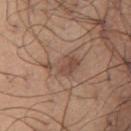Impression:
The lesion was photographed on a routine skin check and not biopsied; there is no pathology result.
Clinical summary:
Measured at roughly 4 mm in maximum diameter. The lesion is on the chest. The patient is a male aged 73–77. Captured under white-light illumination. A close-up tile cropped from a whole-body skin photograph, about 15 mm across.